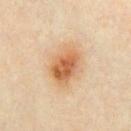follow-up = no biopsy performed (imaged during a skin exam)
imaging modality = ~15 mm crop, total-body skin-cancer survey
body site = the chest
lesion diameter = ~6 mm (longest diameter)
lighting = cross-polarized illumination
patient = male, about 35 years old